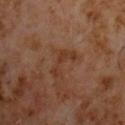Recorded during total-body skin imaging; not selected for excision or biopsy.
A 15 mm close-up extracted from a 3D total-body photography capture.
Imaged with cross-polarized lighting.
The subject is a male in their 60s.
The lesion's longest dimension is about 4.5 mm.
On the chest.